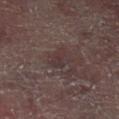This lesion was catalogued during total-body skin photography and was not selected for biopsy. Measured at roughly 3 mm in maximum diameter. Automated image analysis of the tile measured a symmetry-axis asymmetry near 0.35. The software also gave a lesion color around L≈28 a*≈13 b*≈12 in CIELAB, a lesion–skin lightness drop of about 5, and a normalized lesion–skin contrast near 5.5. The analysis additionally found a border-irregularity index near 3.5/10, internal color variation of about 1 on a 0–10 scale, and peripheral color asymmetry of about 0.5. The software also gave a classifier nevus-likeness of about 0/100. Imaged with cross-polarized lighting. Cropped from a total-body skin-imaging series; the visible field is about 15 mm. A male patient aged 58–62. The lesion is located on the left lower leg.